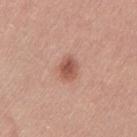Impression:
No biopsy was performed on this lesion — it was imaged during a full skin examination and was not determined to be concerning.
Image and clinical context:
Captured under white-light illumination. The lesion is on the left thigh. A female subject about 25 years old. This image is a 15 mm lesion crop taken from a total-body photograph.